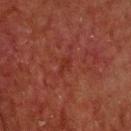The lesion was tiled from a total-body skin photograph and was not biopsied. A male patient, approximately 70 years of age. Approximately 2.5 mm at its widest. Cropped from a total-body skin-imaging series; the visible field is about 15 mm. Imaged with cross-polarized lighting. On the head or neck.